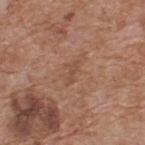Q: What did automated image analysis measure?
A: an eccentricity of roughly 0.9; an automated nevus-likeness rating near 0 out of 100 and a lesion-detection confidence of about 100/100
Q: What kind of image is this?
A: 15 mm crop, total-body photography
Q: What is the anatomic site?
A: the upper back
Q: Illumination type?
A: white-light illumination
Q: Who is the patient?
A: male, in their 60s